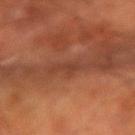Imaged during a routine full-body skin examination; the lesion was not biopsied and no histopathology is available. The tile uses cross-polarized illumination. The lesion's longest dimension is about 2.5 mm. The lesion is on the right forearm. A male patient about 55 years old. This image is a 15 mm lesion crop taken from a total-body photograph.